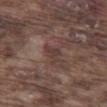Captured during whole-body skin photography for melanoma surveillance; the lesion was not biopsied.
Imaged with white-light lighting.
This image is a 15 mm lesion crop taken from a total-body photograph.
On the left thigh.
The lesion's longest dimension is about 3 mm.
The lesion-visualizer software estimated an eccentricity of roughly 0.8. It also reported a mean CIELAB color near L≈37 a*≈17 b*≈19 and roughly 7 lightness units darker than nearby skin. The analysis additionally found a border-irregularity rating of about 4.5/10 and a color-variation rating of about 1/10.
A male subject, aged 73–77.